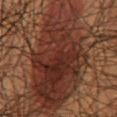size: ≈13 mm | image-analysis metrics: an area of roughly 70 mm²; a lesion color around L≈22 a*≈17 b*≈20 in CIELAB, a lesion–skin lightness drop of about 8, and a normalized border contrast of about 9.5; a border-irregularity rating of about 5.5/10; a nevus-likeness score of about 85/100 and a detector confidence of about 90 out of 100 that the crop contains a lesion | location: the back | illumination: cross-polarized | imaging modality: ~15 mm crop, total-body skin-cancer survey | subject: male, in their 50s.